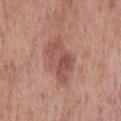Impression: Part of a total-body skin-imaging series; this lesion was reviewed on a skin check and was not flagged for biopsy. Acquisition and patient details: A 15 mm close-up extracted from a 3D total-body photography capture. The tile uses white-light illumination. Located on the mid back. The patient is a male aged approximately 60.